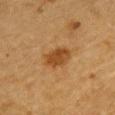biopsy_status: not biopsied; imaged during a skin examination
lighting: cross-polarized
patient:
  sex: male
  age_approx: 85+
site: chest
image:
  source: total-body photography crop
  field_of_view_mm: 15
lesion_size:
  long_diameter_mm_approx: 3.5